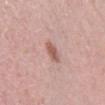Impression:
Recorded during total-body skin imaging; not selected for excision or biopsy.
Acquisition and patient details:
Longest diameter approximately 2.5 mm. Imaged with white-light lighting. The lesion is on the right lower leg. A lesion tile, about 15 mm wide, cut from a 3D total-body photograph. A female subject approximately 40 years of age. Automated tile analysis of the lesion measured roughly 11 lightness units darker than nearby skin and a lesion-to-skin contrast of about 7.5 (normalized; higher = more distinct).